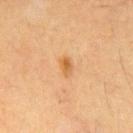- workup: total-body-photography surveillance lesion; no biopsy
- location: the chest
- patient: male, approximately 60 years of age
- image-analysis metrics: an area of roughly 3 mm², a shape eccentricity near 0.8, and a shape-asymmetry score of about 0.25 (0 = symmetric); a border-irregularity index near 2.5/10 and radial color variation of about 1.5; a classifier nevus-likeness of about 65/100 and a lesion-detection confidence of about 100/100
- imaging modality: 15 mm crop, total-body photography
- diameter: ≈2.5 mm